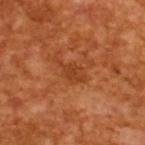This lesion was catalogued during total-body skin photography and was not selected for biopsy. The total-body-photography lesion software estimated a lesion-to-skin contrast of about 5.5 (normalized; higher = more distinct). About 4 mm across. A male patient aged 63–67. Cropped from a total-body skin-imaging series; the visible field is about 15 mm. This is a cross-polarized tile.